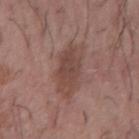Recorded during total-body skin imaging; not selected for excision or biopsy. A male subject aged approximately 60. Cropped from a total-body skin-imaging series; the visible field is about 15 mm. On the right forearm.